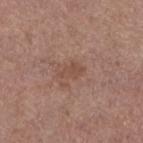The lesion was photographed on a routine skin check and not biopsied; there is no pathology result. A female patient in their mid- to late 60s. Approximately 3 mm at its widest. A close-up tile cropped from a whole-body skin photograph, about 15 mm across. The lesion is on the left lower leg.